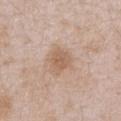{
  "biopsy_status": "not biopsied; imaged during a skin examination",
  "lesion_size": {
    "long_diameter_mm_approx": 3.5
  },
  "site": "front of the torso",
  "patient": {
    "sex": "male",
    "age_approx": 50
  },
  "lighting": "white-light",
  "image": {
    "source": "total-body photography crop",
    "field_of_view_mm": 15
  },
  "automated_metrics": {
    "cielab_L": 59,
    "cielab_a": 17,
    "cielab_b": 30,
    "vs_skin_darker_L": 9.0,
    "vs_skin_contrast_norm": 6.5,
    "border_irregularity_0_10": 2.5,
    "nevus_likeness_0_100": 25,
    "lesion_detection_confidence_0_100": 100
  }
}